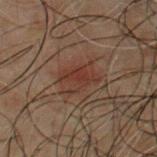Assessment:
The lesion was photographed on a routine skin check and not biopsied; there is no pathology result.
Clinical summary:
The subject is a male aged around 50. This is a cross-polarized tile. A 15 mm close-up extracted from a 3D total-body photography capture. The recorded lesion diameter is about 4 mm. From the chest. Automated image analysis of the tile measured an outline eccentricity of about 0.75 (0 = round, 1 = elongated) and a symmetry-axis asymmetry near 0.2. And it measured a border-irregularity rating of about 2.5/10, internal color variation of about 3.5 on a 0–10 scale, and peripheral color asymmetry of about 1.